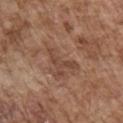Q: Is there a histopathology result?
A: total-body-photography surveillance lesion; no biopsy
Q: Lesion location?
A: the chest
Q: What is the lesion's diameter?
A: ~4.5 mm (longest diameter)
Q: What are the patient's age and sex?
A: male, in their mid- to late 70s
Q: What did automated image analysis measure?
A: a lesion area of about 4 mm² and a symmetry-axis asymmetry near 0.75; a lesion color around L≈44 a*≈20 b*≈28 in CIELAB, roughly 8 lightness units darker than nearby skin, and a lesion-to-skin contrast of about 6 (normalized; higher = more distinct); a border-irregularity rating of about 8.5/10, a within-lesion color-variation index near 0/10, and radial color variation of about 0
Q: How was this image acquired?
A: 15 mm crop, total-body photography
Q: How was the tile lit?
A: white-light illumination